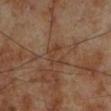| feature | finding |
|---|---|
| biopsy status | catalogued during a skin exam; not biopsied |
| subject | male, roughly 70 years of age |
| lighting | cross-polarized illumination |
| imaging modality | total-body-photography crop, ~15 mm field of view |
| body site | the left lower leg |
| automated metrics | roughly 5 lightness units darker than nearby skin and a lesion-to-skin contrast of about 5 (normalized; higher = more distinct); an automated nevus-likeness rating near 0 out of 100 and a detector confidence of about 100 out of 100 that the crop contains a lesion |